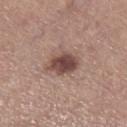Impression: No biopsy was performed on this lesion — it was imaged during a full skin examination and was not determined to be concerning. Background: This is a white-light tile. Approximately 4 mm at its widest. Located on the left lower leg. A female subject aged 63–67. A lesion tile, about 15 mm wide, cut from a 3D total-body photograph.